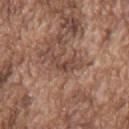Clinical impression:
The lesion was tiled from a total-body skin photograph and was not biopsied.
Image and clinical context:
The tile uses white-light illumination. The lesion's longest dimension is about 2.5 mm. A male patient, aged approximately 75. Located on the mid back. A lesion tile, about 15 mm wide, cut from a 3D total-body photograph. Automated tile analysis of the lesion measured a shape eccentricity near 0.8 and a shape-asymmetry score of about 0.55 (0 = symmetric). The analysis additionally found a lesion color around L≈44 a*≈19 b*≈26 in CIELAB, about 8 CIELAB-L* units darker than the surrounding skin, and a lesion-to-skin contrast of about 6.5 (normalized; higher = more distinct). The software also gave a color-variation rating of about 4/10 and a peripheral color-asymmetry measure near 1.5. The analysis additionally found a classifier nevus-likeness of about 0/100.